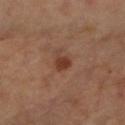The lesion was tiled from a total-body skin photograph and was not biopsied. A 15 mm crop from a total-body photograph taken for skin-cancer surveillance. On the right upper arm. The subject is about 65 years old.